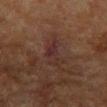follow-up = no biopsy performed (imaged during a skin exam); image source = ~15 mm tile from a whole-body skin photo; location = the right forearm; patient = female, aged approximately 80.